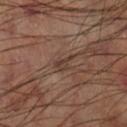The lesion was tiled from a total-body skin photograph and was not biopsied.
Cropped from a total-body skin-imaging series; the visible field is about 15 mm.
The lesion is on the leg.